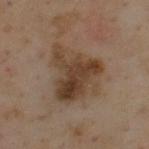Q: Was a biopsy performed?
A: total-body-photography surveillance lesion; no biopsy
Q: How large is the lesion?
A: ≈6.5 mm
Q: Who is the patient?
A: male, in their mid-50s
Q: What did automated image analysis measure?
A: a footprint of about 25 mm², an eccentricity of roughly 0.45, and a shape-asymmetry score of about 0.35 (0 = symmetric); roughly 10 lightness units darker than nearby skin and a lesion-to-skin contrast of about 8.5 (normalized; higher = more distinct)
Q: How was this image acquired?
A: ~15 mm crop, total-body skin-cancer survey
Q: Lesion location?
A: the upper back
Q: What lighting was used for the tile?
A: cross-polarized illumination